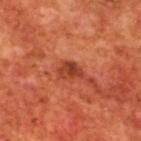biopsy status=imaged on a skin check; not biopsied.